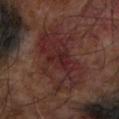<tbp_lesion>
<biopsy_status>not biopsied; imaged during a skin examination</biopsy_status>
<automated_metrics>
  <area_mm2_approx>32.0</area_mm2_approx>
  <eccentricity>0.8</eccentricity>
  <shape_asymmetry>0.2</shape_asymmetry>
  <cielab_L>26</cielab_L>
  <cielab_a>20</cielab_a>
  <cielab_b>19</cielab_b>
  <vs_skin_darker_L>6.0</vs_skin_darker_L>
  <vs_skin_contrast_norm>7.0</vs_skin_contrast_norm>
  <border_irregularity_0_10>4.0</border_irregularity_0_10>
  <color_variation_0_10>6.0</color_variation_0_10>
  <peripheral_color_asymmetry>2.0</peripheral_color_asymmetry>
  <nevus_likeness_0_100>0</nevus_likeness_0_100>
</automated_metrics>
<image>
  <source>total-body photography crop</source>
  <field_of_view_mm>15</field_of_view_mm>
</image>
<patient>
  <sex>male</sex>
  <age_approx>65</age_approx>
</patient>
<site>left forearm</site>
<lighting>cross-polarized</lighting>
</tbp_lesion>